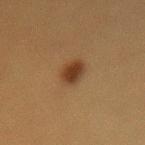| feature | finding |
|---|---|
| follow-up | catalogued during a skin exam; not biopsied |